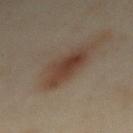This lesion was catalogued during total-body skin photography and was not selected for biopsy. The patient is a female aged around 35. From the mid back. A roughly 15 mm field-of-view crop from a total-body skin photograph. The tile uses cross-polarized illumination.